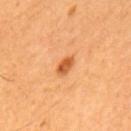biopsy status: imaged on a skin check; not biopsied
automated lesion analysis: an average lesion color of about L≈56 a*≈29 b*≈44 (CIELAB) and a normalized lesion–skin contrast near 8.5
lighting: cross-polarized
imaging modality: total-body-photography crop, ~15 mm field of view
patient: male, about 60 years old
anatomic site: the mid back